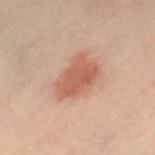Clinical impression: Captured during whole-body skin photography for melanoma surveillance; the lesion was not biopsied. Clinical summary: Cropped from a whole-body photographic skin survey; the tile spans about 15 mm. Measured at roughly 5 mm in maximum diameter. Captured under cross-polarized illumination. On the chest. A female patient, approximately 65 years of age.